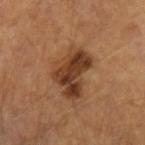Q: Was this lesion biopsied?
A: catalogued during a skin exam; not biopsied
Q: What is the lesion's diameter?
A: ~5.5 mm (longest diameter)
Q: How was this image acquired?
A: ~15 mm crop, total-body skin-cancer survey
Q: Automated lesion metrics?
A: an automated nevus-likeness rating near 60 out of 100 and a detector confidence of about 100 out of 100 that the crop contains a lesion
Q: What is the anatomic site?
A: the left forearm
Q: What are the patient's age and sex?
A: male, in their mid- to late 60s